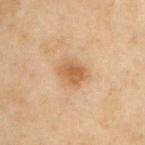Clinical impression: Imaged during a routine full-body skin examination; the lesion was not biopsied and no histopathology is available. Clinical summary: From the chest. Cropped from a total-body skin-imaging series; the visible field is about 15 mm. The patient is a male aged 53 to 57.